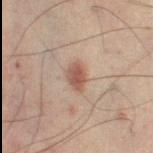Q: Is there a histopathology result?
A: catalogued during a skin exam; not biopsied
Q: What is the lesion's diameter?
A: about 3 mm
Q: Illumination type?
A: cross-polarized
Q: Who is the patient?
A: male, in their 50s
Q: How was this image acquired?
A: total-body-photography crop, ~15 mm field of view
Q: What did automated image analysis measure?
A: a footprint of about 4.5 mm², a shape eccentricity near 0.75, and a symmetry-axis asymmetry near 0.15; a nevus-likeness score of about 95/100 and a detector confidence of about 100 out of 100 that the crop contains a lesion
Q: What is the anatomic site?
A: the right lower leg